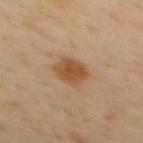Clinical impression:
The lesion was tiled from a total-body skin photograph and was not biopsied.
Background:
Cropped from a total-body skin-imaging series; the visible field is about 15 mm. The patient is a male aged 38 to 42. This is a cross-polarized tile. The recorded lesion diameter is about 3.5 mm. On the back.